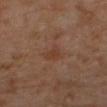Captured during whole-body skin photography for melanoma surveillance; the lesion was not biopsied. A region of skin cropped from a whole-body photographic capture, roughly 15 mm wide. Imaged with cross-polarized lighting. On the left lower leg. Approximately 2.5 mm at its widest. A male patient, roughly 30 years of age.